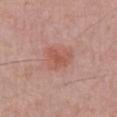| field | value |
|---|---|
| follow-up | imaged on a skin check; not biopsied |
| location | the chest |
| patient | male, about 65 years old |
| illumination | white-light illumination |
| acquisition | total-body-photography crop, ~15 mm field of view |